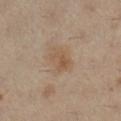Part of a total-body skin-imaging series; this lesion was reviewed on a skin check and was not flagged for biopsy. Captured under cross-polarized illumination. Automated tile analysis of the lesion measured a shape eccentricity near 0.75 and a symmetry-axis asymmetry near 0.2. It also reported a border-irregularity index near 2/10, internal color variation of about 4 on a 0–10 scale, and a peripheral color-asymmetry measure near 1.5. The analysis additionally found a detector confidence of about 100 out of 100 that the crop contains a lesion. The lesion is on the left leg. The subject is a female about 40 years old. A region of skin cropped from a whole-body photographic capture, roughly 15 mm wide. Measured at roughly 3 mm in maximum diameter.